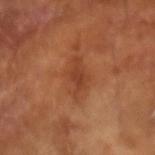<tbp_lesion>
  <biopsy_status>not biopsied; imaged during a skin examination</biopsy_status>
  <lesion_size>
    <long_diameter_mm_approx>5.0</long_diameter_mm_approx>
  </lesion_size>
  <automated_metrics>
    <cielab_L>41</cielab_L>
    <cielab_a>25</cielab_a>
    <cielab_b>34</cielab_b>
    <vs_skin_darker_L>7.0</vs_skin_darker_L>
    <vs_skin_contrast_norm>6.0</vs_skin_contrast_norm>
    <border_irregularity_0_10>4.5</border_irregularity_0_10>
    <nevus_likeness_0_100>0</nevus_likeness_0_100>
    <lesion_detection_confidence_0_100>100</lesion_detection_confidence_0_100>
  </automated_metrics>
  <image>
    <source>total-body photography crop</source>
    <field_of_view_mm>15</field_of_view_mm>
  </image>
  <patient>
    <sex>male</sex>
    <age_approx>65</age_approx>
  </patient>
</tbp_lesion>